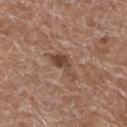Impression: Captured during whole-body skin photography for melanoma surveillance; the lesion was not biopsied. Acquisition and patient details: The lesion's longest dimension is about 4 mm. A male subject, roughly 75 years of age. Imaged with white-light lighting. A 15 mm close-up tile from a total-body photography series done for melanoma screening. The lesion is on the left lower leg.